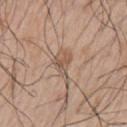Imaged during a routine full-body skin examination; the lesion was not biopsied and no histopathology is available. Automated image analysis of the tile measured an area of roughly 6 mm² and two-axis asymmetry of about 0.45. And it measured a mean CIELAB color near L≈55 a*≈16 b*≈28. And it measured a border-irregularity index near 5/10 and a color-variation rating of about 4.5/10. And it measured a classifier nevus-likeness of about 0/100 and a lesion-detection confidence of about 60/100. This is a white-light tile. Cropped from a whole-body photographic skin survey; the tile spans about 15 mm. The recorded lesion diameter is about 4.5 mm. A male patient about 65 years old. On the left upper arm.